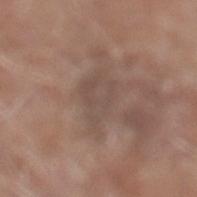| key | value |
|---|---|
| biopsy status | total-body-photography surveillance lesion; no biopsy |
| acquisition | total-body-photography crop, ~15 mm field of view |
| TBP lesion metrics | an area of roughly 9.5 mm² and an outline eccentricity of about 0.8 (0 = round, 1 = elongated); border irregularity of about 4.5 on a 0–10 scale and a color-variation rating of about 2/10; a nevus-likeness score of about 0/100 and a lesion-detection confidence of about 80/100 |
| site | the left lower leg |
| lesion diameter | ≈4.5 mm |
| patient | male, aged 78 to 82 |
| tile lighting | white-light |